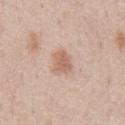| feature | finding |
|---|---|
| biopsy status | imaged on a skin check; not biopsied |
| size | about 3 mm |
| image source | 15 mm crop, total-body photography |
| location | the chest |
| patient | male, approximately 70 years of age |